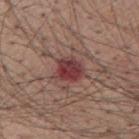The lesion was tiled from a total-body skin photograph and was not biopsied. A male patient about 60 years old. Automated image analysis of the tile measured a mean CIELAB color near L≈38 a*≈26 b*≈20 and about 12 CIELAB-L* units darker than the surrounding skin. It also reported a border-irregularity index near 2/10, internal color variation of about 6.5 on a 0–10 scale, and radial color variation of about 2.5. This is a white-light tile. The lesion is located on the back. A 15 mm crop from a total-body photograph taken for skin-cancer surveillance.